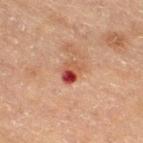Q: Was a biopsy performed?
A: no biopsy performed (imaged during a skin exam)
Q: How large is the lesion?
A: ≈3 mm
Q: Where on the body is the lesion?
A: the leg
Q: How was the tile lit?
A: cross-polarized
Q: How was this image acquired?
A: ~15 mm tile from a whole-body skin photo
Q: Patient demographics?
A: female, aged 53–57